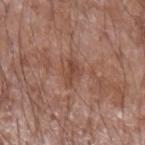Q: Was this lesion biopsied?
A: no biopsy performed (imaged during a skin exam)
Q: Where on the body is the lesion?
A: the left forearm
Q: Patient demographics?
A: male, approximately 60 years of age
Q: How was the tile lit?
A: white-light illumination
Q: What kind of image is this?
A: ~15 mm crop, total-body skin-cancer survey